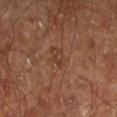Findings:
• biopsy status: total-body-photography surveillance lesion; no biopsy
• diameter: ~2.5 mm (longest diameter)
• patient: male, aged approximately 60
• image: ~15 mm crop, total-body skin-cancer survey
• body site: the right leg
• lighting: cross-polarized